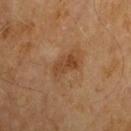follow-up: catalogued during a skin exam; not biopsied | anatomic site: the chest | subject: male, aged 58 to 62 | imaging modality: total-body-photography crop, ~15 mm field of view | automated lesion analysis: a footprint of about 6 mm² and two-axis asymmetry of about 0.25; a border-irregularity rating of about 3/10, a within-lesion color-variation index near 4/10, and peripheral color asymmetry of about 1.5; a classifier nevus-likeness of about 0/100 and a lesion-detection confidence of about 100/100 | diameter: ≈4 mm.